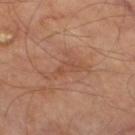A lesion tile, about 15 mm wide, cut from a 3D total-body photograph. The lesion is located on the right thigh. The subject is a male aged approximately 70. The lesion-visualizer software estimated a lesion area of about 8.5 mm² and two-axis asymmetry of about 0.6. It also reported a mean CIELAB color near L≈49 a*≈21 b*≈30 and about 6 CIELAB-L* units darker than the surrounding skin. The analysis additionally found border irregularity of about 8 on a 0–10 scale, internal color variation of about 2 on a 0–10 scale, and a peripheral color-asymmetry measure near 0.5. Approximately 5.5 mm at its widest.